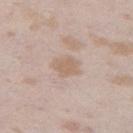The lesion was tiled from a total-body skin photograph and was not biopsied.
Located on the left thigh.
A 15 mm crop from a total-body photograph taken for skin-cancer surveillance.
A female patient, aged 23 to 27.
Longest diameter approximately 3 mm.
Imaged with white-light lighting.